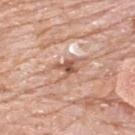Q: Was this lesion biopsied?
A: no biopsy performed (imaged during a skin exam)
Q: How was this image acquired?
A: 15 mm crop, total-body photography
Q: What are the patient's age and sex?
A: male, aged approximately 80
Q: How was the tile lit?
A: white-light illumination
Q: Automated lesion metrics?
A: a lesion color around L≈57 a*≈23 b*≈30 in CIELAB, about 13 CIELAB-L* units darker than the surrounding skin, and a normalized lesion–skin contrast near 8.5; border irregularity of about 5.5 on a 0–10 scale and a color-variation rating of about 3.5/10; a lesion-detection confidence of about 100/100
Q: Lesion location?
A: the back
Q: What is the lesion's diameter?
A: ≈3 mm